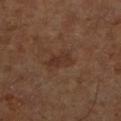Notes:
- follow-up: imaged on a skin check; not biopsied
- subject: approximately 65 years of age
- location: the leg
- image: ~15 mm tile from a whole-body skin photo